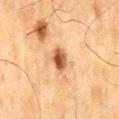The lesion was photographed on a routine skin check and not biopsied; there is no pathology result. This is a cross-polarized tile. The lesion is located on the mid back. A male subject, approximately 70 years of age. A close-up tile cropped from a whole-body skin photograph, about 15 mm across. Approximately 3.5 mm at its widest. Automated image analysis of the tile measured an average lesion color of about L≈48 a*≈21 b*≈34 (CIELAB), a lesion–skin lightness drop of about 15, and a normalized border contrast of about 10.5.